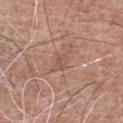Assessment:
Recorded during total-body skin imaging; not selected for excision or biopsy.
Acquisition and patient details:
Located on the chest. Approximately 3.5 mm at its widest. A 15 mm close-up extracted from a 3D total-body photography capture. Automated tile analysis of the lesion measured an area of roughly 4 mm², a shape eccentricity near 0.85, and a symmetry-axis asymmetry near 0.4. The analysis additionally found a lesion–skin lightness drop of about 6. The analysis additionally found a border-irregularity rating of about 4.5/10 and internal color variation of about 1.5 on a 0–10 scale. The subject is a male about 65 years old.